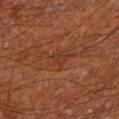The lesion was tiled from a total-body skin photograph and was not biopsied.
This is a cross-polarized tile.
The total-body-photography lesion software estimated a border-irregularity rating of about 4.5/10 and internal color variation of about 1.5 on a 0–10 scale. The software also gave lesion-presence confidence of about 95/100.
A roughly 15 mm field-of-view crop from a total-body skin photograph.
The lesion is on the left lower leg.
Approximately 2.5 mm at its widest.
A male subject in their mid- to late 60s.